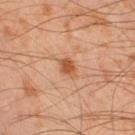  biopsy_status: not biopsied; imaged during a skin examination
  image:
    source: total-body photography crop
    field_of_view_mm: 15
  site: left lower leg
  lighting: cross-polarized
  patient:
    sex: male
    age_approx: 45